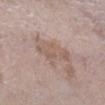Impression: The lesion was tiled from a total-body skin photograph and was not biopsied. Clinical summary: A lesion tile, about 15 mm wide, cut from a 3D total-body photograph. A male subject, aged 58–62. Located on the right lower leg.